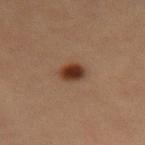No biopsy was performed on this lesion — it was imaged during a full skin examination and was not determined to be concerning. The recorded lesion diameter is about 2.5 mm. On the abdomen. This is a cross-polarized tile. A female patient, approximately 40 years of age. Cropped from a total-body skin-imaging series; the visible field is about 15 mm.